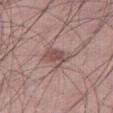– follow-up — no biopsy performed (imaged during a skin exam)
– patient — male, aged around 60
– diameter — ≈3.5 mm
– imaging modality — total-body-photography crop, ~15 mm field of view
– body site — the right thigh
– image-analysis metrics — a lesion area of about 6.5 mm², a shape eccentricity near 0.7, and a shape-asymmetry score of about 0.2 (0 = symmetric); about 10 CIELAB-L* units darker than the surrounding skin and a lesion-to-skin contrast of about 7 (normalized; higher = more distinct); a border-irregularity rating of about 3/10, a color-variation rating of about 3/10, and radial color variation of about 1; a classifier nevus-likeness of about 10/100
– tile lighting — white-light